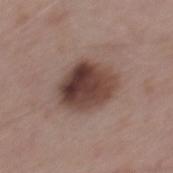Recorded during total-body skin imaging; not selected for excision or biopsy.
Measured at roughly 5.5 mm in maximum diameter.
The tile uses white-light illumination.
A roughly 15 mm field-of-view crop from a total-body skin photograph.
A male patient, in their mid- to late 50s.
Automated image analysis of the tile measured an eccentricity of roughly 0.55. The analysis additionally found a lesion color around L≈41 a*≈18 b*≈22 in CIELAB and a normalized lesion–skin contrast near 11.5.